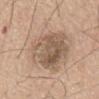lighting: white-light
imaging modality: total-body-photography crop, ~15 mm field of view
anatomic site: the back
patient: male, aged 63 to 67
automated metrics: a footprint of about 44 mm² and a shape-asymmetry score of about 0.15 (0 = symmetric); roughly 10 lightness units darker than nearby skin and a lesion-to-skin contrast of about 6.5 (normalized; higher = more distinct)
lesion size: about 9.5 mm